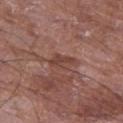From the left thigh. Imaged with white-light lighting. A close-up tile cropped from a whole-body skin photograph, about 15 mm across. The lesion's longest dimension is about 3.5 mm. The patient is a male aged 63–67.